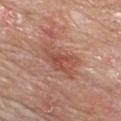Assessment:
Recorded during total-body skin imaging; not selected for excision or biopsy.
Acquisition and patient details:
About 5.5 mm across. A female subject aged 68–72. Imaged with white-light lighting. The lesion is on the chest. A 15 mm crop from a total-body photograph taken for skin-cancer surveillance.